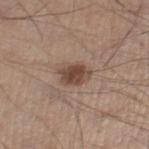follow-up: no biopsy performed (imaged during a skin exam)
subject: male, in their mid- to late 40s
image source: ~15 mm crop, total-body skin-cancer survey
site: the right thigh